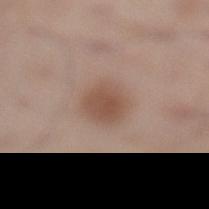Imaged during a routine full-body skin examination; the lesion was not biopsied and no histopathology is available. On the leg. A male subject aged 58–62. A 15 mm crop from a total-body photograph taken for skin-cancer surveillance. About 3.5 mm across.